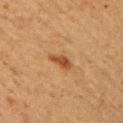Impression:
No biopsy was performed on this lesion — it was imaged during a full skin examination and was not determined to be concerning.
Acquisition and patient details:
Captured under cross-polarized illumination. A 15 mm close-up extracted from a 3D total-body photography capture. The recorded lesion diameter is about 3 mm. From the right upper arm. A female patient roughly 60 years of age.